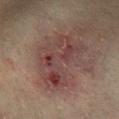Acquisition and patient details:
A male subject, aged approximately 65. Located on the leg. Captured under cross-polarized illumination. A roughly 15 mm field-of-view crop from a total-body skin photograph.